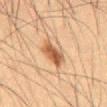Image and clinical context:
The lesion is located on the abdomen. A 15 mm close-up extracted from a 3D total-body photography capture. A male patient aged approximately 50. The tile uses cross-polarized illumination. Approximately 4 mm at its widest.